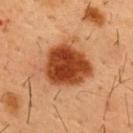Q: What lighting was used for the tile?
A: cross-polarized illumination
Q: What are the patient's age and sex?
A: male, in their mid-50s
Q: What is the anatomic site?
A: the front of the torso
Q: How was this image acquired?
A: ~15 mm crop, total-body skin-cancer survey